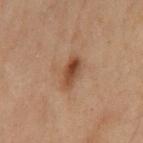workup: no biopsy performed (imaged during a skin exam) | location: the chest | automated lesion analysis: a lesion area of about 5 mm², a shape eccentricity near 0.9, and a symmetry-axis asymmetry near 0.25; a lesion color around L≈34 a*≈17 b*≈26 in CIELAB, about 10 CIELAB-L* units darker than the surrounding skin, and a normalized border contrast of about 9; border irregularity of about 3 on a 0–10 scale, a color-variation rating of about 3.5/10, and radial color variation of about 1.5; a classifier nevus-likeness of about 95/100 and a detector confidence of about 100 out of 100 that the crop contains a lesion | imaging modality: 15 mm crop, total-body photography | size: about 4 mm | patient: male, approximately 70 years of age | illumination: cross-polarized illumination.